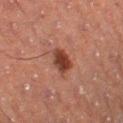<record>
<biopsy_status>not biopsied; imaged during a skin examination</biopsy_status>
<image>
  <source>total-body photography crop</source>
  <field_of_view_mm>15</field_of_view_mm>
</image>
<automated_metrics>
  <area_mm2_approx>5.5</area_mm2_approx>
  <eccentricity>0.65</eccentricity>
  <shape_asymmetry>0.25</shape_asymmetry>
  <cielab_L>34</cielab_L>
  <cielab_a>23</cielab_a>
  <cielab_b>26</cielab_b>
  <vs_skin_contrast_norm>10.5</vs_skin_contrast_norm>
  <border_irregularity_0_10>2.0</border_irregularity_0_10>
  <color_variation_0_10>4.5</color_variation_0_10>
  <peripheral_color_asymmetry>1.5</peripheral_color_asymmetry>
  <nevus_likeness_0_100>95</nevus_likeness_0_100>
  <lesion_detection_confidence_0_100>100</lesion_detection_confidence_0_100>
</automated_metrics>
<patient>
  <sex>male</sex>
  <age_approx>55</age_approx>
</patient>
<site>leg</site>
<lighting>cross-polarized</lighting>
</record>